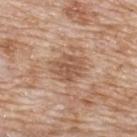Assessment: Imaged during a routine full-body skin examination; the lesion was not biopsied and no histopathology is available. Clinical summary: The lesion is on the upper back. A male subject roughly 80 years of age. The total-body-photography lesion software estimated a normalized border contrast of about 7. The analysis additionally found a border-irregularity index near 2/10 and a within-lesion color-variation index near 2.5/10. The tile uses white-light illumination. A 15 mm close-up tile from a total-body photography series done for melanoma screening. The recorded lesion diameter is about 3.5 mm.